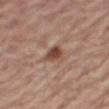follow-up — total-body-photography surveillance lesion; no biopsy
patient — male, in their 80s
image source — ~15 mm crop, total-body skin-cancer survey
location — the right thigh
diameter — ≈2.5 mm